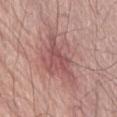Part of a total-body skin-imaging series; this lesion was reviewed on a skin check and was not flagged for biopsy.
A close-up tile cropped from a whole-body skin photograph, about 15 mm across.
About 9 mm across.
A male subject aged approximately 80.
Captured under white-light illumination.
From the abdomen.
The total-body-photography lesion software estimated an area of roughly 28 mm² and a symmetry-axis asymmetry near 0.35. The software also gave about 9 CIELAB-L* units darker than the surrounding skin and a normalized lesion–skin contrast near 6.5. The software also gave a border-irregularity index near 5.5/10, a color-variation rating of about 5.5/10, and peripheral color asymmetry of about 2.